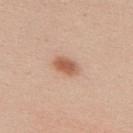Imaged during a routine full-body skin examination; the lesion was not biopsied and no histopathology is available.
Captured under white-light illumination.
A roughly 15 mm field-of-view crop from a total-body skin photograph.
The patient is a female about 20 years old.
Approximately 3 mm at its widest.
The lesion is located on the back.